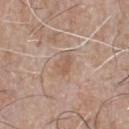Captured during whole-body skin photography for melanoma surveillance; the lesion was not biopsied.